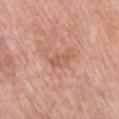Q: Was a biopsy performed?
A: catalogued during a skin exam; not biopsied
Q: What is the anatomic site?
A: the chest
Q: What did automated image analysis measure?
A: an outline eccentricity of about 0.9 (0 = round, 1 = elongated) and a shape-asymmetry score of about 0.45 (0 = symmetric); a border-irregularity index near 5.5/10, a within-lesion color-variation index near 0/10, and peripheral color asymmetry of about 0
Q: What kind of image is this?
A: ~15 mm tile from a whole-body skin photo
Q: What are the patient's age and sex?
A: male, aged 78 to 82
Q: What is the lesion's diameter?
A: ≈2.5 mm